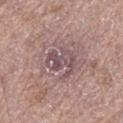Recorded during total-body skin imaging; not selected for excision or biopsy. The lesion is on the left thigh. The patient is a male aged approximately 70. Longest diameter approximately 4 mm. Cropped from a whole-body photographic skin survey; the tile spans about 15 mm.